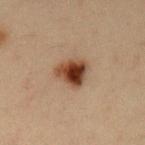Q: Was a biopsy performed?
A: catalogued during a skin exam; not biopsied
Q: Illumination type?
A: cross-polarized illumination
Q: How was this image acquired?
A: 15 mm crop, total-body photography
Q: How large is the lesion?
A: ≈3.5 mm
Q: Lesion location?
A: the left upper arm
Q: What are the patient's age and sex?
A: male, aged around 35